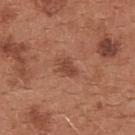Q: Was this lesion biopsied?
A: total-body-photography surveillance lesion; no biopsy
Q: How was this image acquired?
A: ~15 mm crop, total-body skin-cancer survey
Q: What are the patient's age and sex?
A: female, in their mid- to late 30s
Q: Where on the body is the lesion?
A: the front of the torso